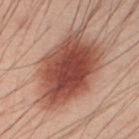The lesion is on the abdomen.
A 15 mm close-up extracted from a 3D total-body photography capture.
A male patient, roughly 40 years of age.
Approximately 9.5 mm at its widest.
The tile uses cross-polarized illumination.